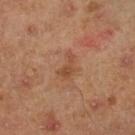Findings:
- workup: catalogued during a skin exam; not biopsied
- imaging modality: ~15 mm tile from a whole-body skin photo
- lighting: cross-polarized
- anatomic site: the leg
- diameter: ≈3 mm
- subject: male, approximately 45 years of age